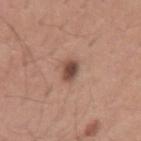No biopsy was performed on this lesion — it was imaged during a full skin examination and was not determined to be concerning.
Measured at roughly 2.5 mm in maximum diameter.
Cropped from a total-body skin-imaging series; the visible field is about 15 mm.
From the left upper arm.
A male subject about 30 years old.
Captured under white-light illumination.